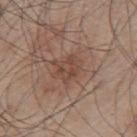{
  "biopsy_status": "not biopsied; imaged during a skin examination",
  "lighting": "white-light",
  "patient": {
    "sex": "male",
    "age_approx": 55
  },
  "site": "mid back",
  "image": {
    "source": "total-body photography crop",
    "field_of_view_mm": 15
  },
  "automated_metrics": {
    "area_mm2_approx": 5.0,
    "eccentricity": 0.75,
    "vs_skin_darker_L": 7.0,
    "vs_skin_contrast_norm": 6.0,
    "nevus_likeness_0_100": 0
  }
}